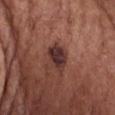<lesion>
  <biopsy_status>not biopsied; imaged during a skin examination</biopsy_status>
  <lesion_size>
    <long_diameter_mm_approx>3.5</long_diameter_mm_approx>
  </lesion_size>
  <lighting>white-light</lighting>
  <image>
    <source>total-body photography crop</source>
    <field_of_view_mm>15</field_of_view_mm>
  </image>
  <site>chest</site>
  <patient>
    <sex>female</sex>
    <age_approx>80</age_approx>
  </patient>
</lesion>